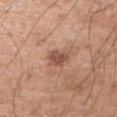Clinical impression:
Recorded during total-body skin imaging; not selected for excision or biopsy.
Background:
A roughly 15 mm field-of-view crop from a total-body skin photograph. This is a white-light tile. Approximately 3 mm at its widest. On the arm. The subject is a male in their mid- to late 50s.